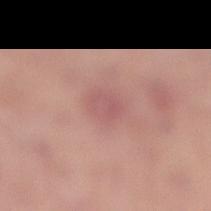Q: Is there a histopathology result?
A: imaged on a skin check; not biopsied
Q: What did automated image analysis measure?
A: an eccentricity of roughly 0.65 and two-axis asymmetry of about 0.2; a mean CIELAB color near L≈57 a*≈25 b*≈22; a classifier nevus-likeness of about 0/100 and lesion-presence confidence of about 100/100
Q: How large is the lesion?
A: ≈3 mm
Q: How was the tile lit?
A: white-light
Q: Where on the body is the lesion?
A: the right lower leg
Q: What is the imaging modality?
A: ~15 mm crop, total-body skin-cancer survey
Q: Patient demographics?
A: female, in their 60s